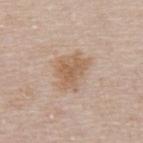The lesion was tiled from a total-body skin photograph and was not biopsied. A female patient approximately 40 years of age. Measured at roughly 4.5 mm in maximum diameter. Cropped from a total-body skin-imaging series; the visible field is about 15 mm. The lesion is located on the upper back. This is a white-light tile.